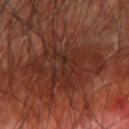{"biopsy_status": "not biopsied; imaged during a skin examination"}